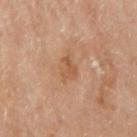biopsy status = no biopsy performed (imaged during a skin exam) | anatomic site = the right upper arm | automated metrics = a lesion color around L≈54 a*≈21 b*≈35 in CIELAB, about 8 CIELAB-L* units darker than the surrounding skin, and a normalized lesion–skin contrast near 6 | size = about 3.5 mm | patient = female, aged around 65 | tile lighting = cross-polarized illumination | acquisition = ~15 mm tile from a whole-body skin photo.